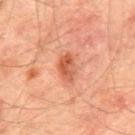Acquisition and patient details: The tile uses cross-polarized illumination. About 3.5 mm across. Located on the mid back. The subject is a male in their mid-60s. Automated image analysis of the tile measured a mean CIELAB color near L≈57 a*≈31 b*≈37, a lesion–skin lightness drop of about 12, and a lesion-to-skin contrast of about 8 (normalized; higher = more distinct). The software also gave a border-irregularity index near 3/10, a color-variation rating of about 4.5/10, and radial color variation of about 1.5. A 15 mm close-up tile from a total-body photography series done for melanoma screening.